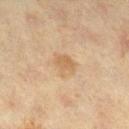Clinical impression:
No biopsy was performed on this lesion — it was imaged during a full skin examination and was not determined to be concerning.
Clinical summary:
A 15 mm crop from a total-body photograph taken for skin-cancer surveillance. The lesion's longest dimension is about 3 mm. A female subject aged 38–42. Automated image analysis of the tile measured a footprint of about 5.5 mm², an outline eccentricity of about 0.55 (0 = round, 1 = elongated), and a symmetry-axis asymmetry near 0.25. The software also gave a mean CIELAB color near L≈54 a*≈14 b*≈33, about 7 CIELAB-L* units darker than the surrounding skin, and a normalized lesion–skin contrast near 5.5. The analysis additionally found a within-lesion color-variation index near 2/10. The analysis additionally found an automated nevus-likeness rating near 10 out of 100 and lesion-presence confidence of about 100/100. The lesion is on the leg. The tile uses cross-polarized illumination.